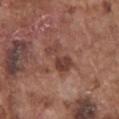Clinical impression: Captured during whole-body skin photography for melanoma surveillance; the lesion was not biopsied. Acquisition and patient details: A male subject roughly 75 years of age. Longest diameter approximately 4 mm. From the chest. A close-up tile cropped from a whole-body skin photograph, about 15 mm across.